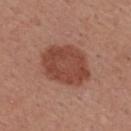notes: catalogued during a skin exam; not biopsied
acquisition: ~15 mm tile from a whole-body skin photo
size: ≈6 mm
anatomic site: the upper back
subject: male, aged approximately 55
automated metrics: a lesion area of about 22 mm²; an average lesion color of about L≈45 a*≈25 b*≈28 (CIELAB) and a normalized lesion–skin contrast near 8.5; a border-irregularity rating of about 1.5/10, a color-variation rating of about 2.5/10, and a peripheral color-asymmetry measure near 1